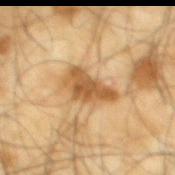biopsy_status: not biopsied; imaged during a skin examination
automated_metrics:
  color_variation_0_10: 4.0
  peripheral_color_asymmetry: 1.5
lighting: cross-polarized
image:
  source: total-body photography crop
  field_of_view_mm: 15
site: mid back
patient:
  sex: male
  age_approx: 65
lesion_size:
  long_diameter_mm_approx: 5.0